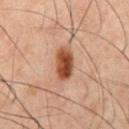Imaged during a routine full-body skin examination; the lesion was not biopsied and no histopathology is available.
A male patient, aged 58 to 62.
From the abdomen.
This is a cross-polarized tile.
This image is a 15 mm lesion crop taken from a total-body photograph.